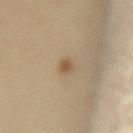Q: What are the patient's age and sex?
A: female, aged around 50
Q: Lesion location?
A: the lower back
Q: What kind of image is this?
A: ~15 mm crop, total-body skin-cancer survey
Q: How large is the lesion?
A: ~2.5 mm (longest diameter)
Q: Illumination type?
A: cross-polarized